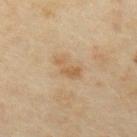| key | value |
|---|---|
| follow-up | imaged on a skin check; not biopsied |
| acquisition | ~15 mm tile from a whole-body skin photo |
| body site | the upper back |
| subject | female, aged 33 to 37 |
| diameter | about 3.5 mm |
| tile lighting | cross-polarized illumination |